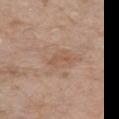Assessment: Recorded during total-body skin imaging; not selected for excision or biopsy. Context: A region of skin cropped from a whole-body photographic capture, roughly 15 mm wide. This is a white-light tile. The lesion is located on the right upper arm. A female subject aged around 85 or older.